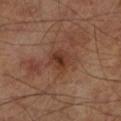workup: imaged on a skin check; not biopsied
subject: male, aged 63–67
diameter: about 3 mm
image: ~15 mm crop, total-body skin-cancer survey